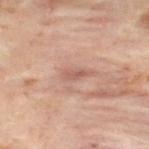Assessment: Captured during whole-body skin photography for melanoma surveillance; the lesion was not biopsied. Image and clinical context: Automated image analysis of the tile measured a lesion area of about 3 mm² and an outline eccentricity of about 0.9 (0 = round, 1 = elongated). The analysis additionally found a border-irregularity rating of about 2.5/10, internal color variation of about 0.5 on a 0–10 scale, and radial color variation of about 0. A male patient, aged 48 to 52. The tile uses cross-polarized illumination. Approximately 2.5 mm at its widest. Cropped from a total-body skin-imaging series; the visible field is about 15 mm. Located on the upper back.